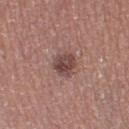workup = no biopsy performed (imaged during a skin exam)
diameter = ~3 mm (longest diameter)
patient = female, aged around 40
site = the leg
image source = ~15 mm crop, total-body skin-cancer survey
tile lighting = white-light